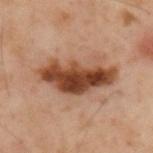Imaged during a routine full-body skin examination; the lesion was not biopsied and no histopathology is available. Measured at roughly 8.5 mm in maximum diameter. The lesion is on the upper back. The patient is a male aged approximately 55. The total-body-photography lesion software estimated a lesion area of about 22 mm², an eccentricity of roughly 0.9, and a shape-asymmetry score of about 0.3 (0 = symmetric). It also reported a normalized border contrast of about 12. The software also gave a border-irregularity rating of about 4.5/10, a within-lesion color-variation index near 8.5/10, and a peripheral color-asymmetry measure near 3. The analysis additionally found a classifier nevus-likeness of about 85/100 and a lesion-detection confidence of about 100/100. A close-up tile cropped from a whole-body skin photograph, about 15 mm across.